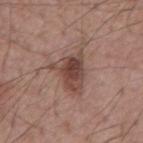Part of a total-body skin-imaging series; this lesion was reviewed on a skin check and was not flagged for biopsy. Located on the mid back. The subject is a male aged around 55. A 15 mm crop from a total-body photograph taken for skin-cancer surveillance.